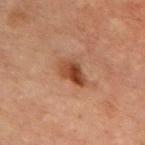No biopsy was performed on this lesion — it was imaged during a full skin examination and was not determined to be concerning.
A 15 mm crop from a total-body photograph taken for skin-cancer surveillance.
Located on the front of the torso.
Approximately 3.5 mm at its widest.
The patient is a male aged approximately 70.